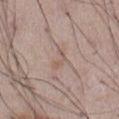Assessment: Imaged during a routine full-body skin examination; the lesion was not biopsied and no histopathology is available. Clinical summary: From the abdomen. A male subject, aged approximately 55. The total-body-photography lesion software estimated an average lesion color of about L≈56 a*≈16 b*≈24 (CIELAB) and a lesion-to-skin contrast of about 5 (normalized; higher = more distinct). And it measured a lesion-detection confidence of about 60/100. The tile uses white-light illumination. A 15 mm close-up extracted from a 3D total-body photography capture. About 2.5 mm across.